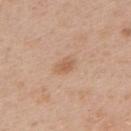This lesion was catalogued during total-body skin photography and was not selected for biopsy. A male patient, aged 33 to 37. On the upper back. Approximately 2.5 mm at its widest. Automated tile analysis of the lesion measured an average lesion color of about L≈60 a*≈19 b*≈33 (CIELAB), about 8 CIELAB-L* units darker than the surrounding skin, and a normalized lesion–skin contrast near 6. The software also gave border irregularity of about 1.5 on a 0–10 scale and radial color variation of about 0.5. Cropped from a total-body skin-imaging series; the visible field is about 15 mm.